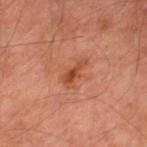Impression: Part of a total-body skin-imaging series; this lesion was reviewed on a skin check and was not flagged for biopsy. Image and clinical context: A male subject, aged 68 to 72. The lesion is on the right lower leg. Imaged with cross-polarized lighting. Measured at roughly 3.5 mm in maximum diameter. An algorithmic analysis of the crop reported an area of roughly 5 mm² and an outline eccentricity of about 0.85 (0 = round, 1 = elongated). And it measured a border-irregularity index near 4.5/10, a color-variation rating of about 2.5/10, and peripheral color asymmetry of about 0.5. A 15 mm close-up tile from a total-body photography series done for melanoma screening.